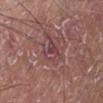<lesion>
  <biopsy_status>not biopsied; imaged during a skin examination</biopsy_status>
  <image>
    <source>total-body photography crop</source>
    <field_of_view_mm>15</field_of_view_mm>
  </image>
  <patient>
    <sex>male</sex>
    <age_approx>55</age_approx>
  </patient>
  <site>left lower leg</site>
</lesion>